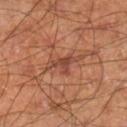| field | value |
|---|---|
| automated lesion analysis | an average lesion color of about L≈43 a*≈25 b*≈29 (CIELAB), roughly 9 lightness units darker than nearby skin, and a normalized lesion–skin contrast near 7; border irregularity of about 6 on a 0–10 scale, a color-variation rating of about 0.5/10, and peripheral color asymmetry of about 0 |
| lesion diameter | ~3 mm (longest diameter) |
| location | the right lower leg |
| patient | male, about 60 years old |
| imaging modality | 15 mm crop, total-body photography |
| tile lighting | cross-polarized illumination |